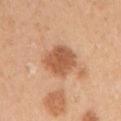Impression: No biopsy was performed on this lesion — it was imaged during a full skin examination and was not determined to be concerning. Background: The total-body-photography lesion software estimated a lesion area of about 13 mm², a shape eccentricity near 0.5, and a symmetry-axis asymmetry near 0.15. The analysis additionally found a border-irregularity index near 2/10, a within-lesion color-variation index near 3/10, and a peripheral color-asymmetry measure near 1. And it measured a nevus-likeness score of about 70/100 and a lesion-detection confidence of about 100/100. Imaged with white-light lighting. The lesion is on the left upper arm. Cropped from a total-body skin-imaging series; the visible field is about 15 mm. The recorded lesion diameter is about 4.5 mm. A female subject about 45 years old.